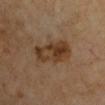Q: Was a biopsy performed?
A: imaged on a skin check; not biopsied
Q: Who is the patient?
A: in their mid- to late 60s
Q: What is the imaging modality?
A: total-body-photography crop, ~15 mm field of view
Q: Where on the body is the lesion?
A: the right upper arm